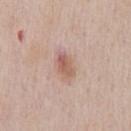{
  "biopsy_status": "not biopsied; imaged during a skin examination",
  "site": "chest",
  "image": {
    "source": "total-body photography crop",
    "field_of_view_mm": 15
  },
  "lighting": "white-light",
  "lesion_size": {
    "long_diameter_mm_approx": 3.0
  },
  "patient": {
    "sex": "male",
    "age_approx": 60
  },
  "automated_metrics": {
    "area_mm2_approx": 4.0,
    "eccentricity": 0.8,
    "shape_asymmetry": 0.2,
    "cielab_L": 58,
    "cielab_a": 21,
    "cielab_b": 27,
    "vs_skin_darker_L": 10.0,
    "vs_skin_contrast_norm": 7.0,
    "border_irregularity_0_10": 2.0,
    "color_variation_0_10": 5.0,
    "peripheral_color_asymmetry": 1.5,
    "nevus_likeness_0_100": 35,
    "lesion_detection_confidence_0_100": 100
  }
}